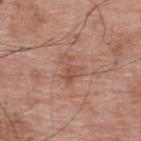<case>
  <biopsy_status>not biopsied; imaged during a skin examination</biopsy_status>
  <patient>
    <sex>male</sex>
    <age_approx>70</age_approx>
  </patient>
  <site>upper back</site>
  <image>
    <source>total-body photography crop</source>
    <field_of_view_mm>15</field_of_view_mm>
  </image>
  <lesion_size>
    <long_diameter_mm_approx>3.5</long_diameter_mm_approx>
  </lesion_size>
  <lighting>white-light</lighting>
</case>